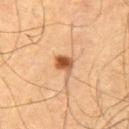biopsy_status: not biopsied; imaged during a skin examination
image:
  source: total-body photography crop
  field_of_view_mm: 15
patient:
  sex: male
  age_approx: 65
automated_metrics:
  area_mm2_approx: 4.0
  eccentricity: 0.5
  shape_asymmetry: 0.35
  cielab_L: 54
  cielab_a: 23
  cielab_b: 38
  vs_skin_contrast_norm: 10.0
site: front of the torso
lesion_size:
  long_diameter_mm_approx: 2.5